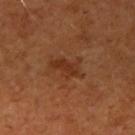- follow-up: total-body-photography surveillance lesion; no biopsy
- acquisition: ~15 mm crop, total-body skin-cancer survey
- illumination: cross-polarized illumination
- patient: female, in their mid-60s
- size: ~4 mm (longest diameter)
- automated metrics: a lesion color around L≈33 a*≈23 b*≈32 in CIELAB, roughly 7 lightness units darker than nearby skin, and a lesion-to-skin contrast of about 6.5 (normalized; higher = more distinct)
- location: the right upper arm